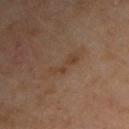{"biopsy_status": "not biopsied; imaged during a skin examination", "image": {"source": "total-body photography crop", "field_of_view_mm": 15}, "lesion_size": {"long_diameter_mm_approx": 4.0}, "patient": {"sex": "male", "age_approx": 70}, "automated_metrics": {"area_mm2_approx": 4.5, "eccentricity": 0.95, "cielab_L": 39, "cielab_a": 16, "cielab_b": 28, "vs_skin_darker_L": 5.0}, "site": "right upper arm", "lighting": "cross-polarized"}